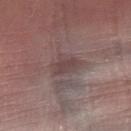biopsy_status: not biopsied; imaged during a skin examination